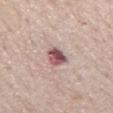{
  "biopsy_status": "not biopsied; imaged during a skin examination",
  "patient": {
    "sex": "male",
    "age_approx": 75
  },
  "image": {
    "source": "total-body photography crop",
    "field_of_view_mm": 15
  },
  "site": "mid back",
  "lighting": "white-light"
}